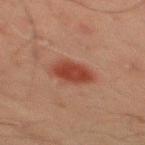Recorded during total-body skin imaging; not selected for excision or biopsy. Captured under cross-polarized illumination. Longest diameter approximately 4 mm. A 15 mm close-up tile from a total-body photography series done for melanoma screening. The lesion is on the mid back. A male subject aged around 45. Automated image analysis of the tile measured a lesion area of about 9 mm² and an outline eccentricity of about 0.7 (0 = round, 1 = elongated). The analysis additionally found a classifier nevus-likeness of about 100/100 and lesion-presence confidence of about 100/100.